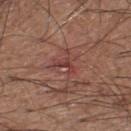biopsy status: total-body-photography surveillance lesion; no biopsy | tile lighting: white-light | automated metrics: a lesion color around L≈40 a*≈27 b*≈23 in CIELAB, about 8 CIELAB-L* units darker than the surrounding skin, and a lesion-to-skin contrast of about 6.5 (normalized; higher = more distinct); a classifier nevus-likeness of about 0/100 | patient: male, in their 60s | imaging modality: ~15 mm tile from a whole-body skin photo | site: the right lower leg | size: about 2.5 mm.